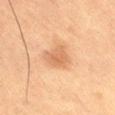Assessment: The lesion was photographed on a routine skin check and not biopsied; there is no pathology result. Image and clinical context: The lesion is located on the leg. This image is a 15 mm lesion crop taken from a total-body photograph. The subject is a male aged 53–57. Captured under cross-polarized illumination. About 4 mm across.